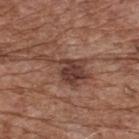This lesion was catalogued during total-body skin photography and was not selected for biopsy. The lesion is on the back. The lesion-visualizer software estimated a footprint of about 11 mm², a shape eccentricity near 0.9, and two-axis asymmetry of about 0.5. The analysis additionally found a border-irregularity rating of about 7/10, a within-lesion color-variation index near 4/10, and radial color variation of about 1.5. And it measured an automated nevus-likeness rating near 40 out of 100 and a detector confidence of about 100 out of 100 that the crop contains a lesion. Cropped from a total-body skin-imaging series; the visible field is about 15 mm. A male patient in their mid- to late 70s. Approximately 6.5 mm at its widest.